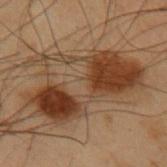Clinical impression: The lesion was photographed on a routine skin check and not biopsied; there is no pathology result. Image and clinical context: Cropped from a whole-body photographic skin survey; the tile spans about 15 mm. A male subject roughly 55 years of age. On the left upper arm.